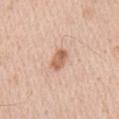<record>
<biopsy_status>not biopsied; imaged during a skin examination</biopsy_status>
<patient>
  <sex>male</sex>
  <age_approx>55</age_approx>
</patient>
<lighting>white-light</lighting>
<site>right upper arm</site>
<lesion_size>
  <long_diameter_mm_approx>3.0</long_diameter_mm_approx>
</lesion_size>
<image>
  <source>total-body photography crop</source>
  <field_of_view_mm>15</field_of_view_mm>
</image>
</record>